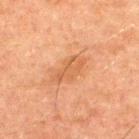The lesion was tiled from a total-body skin photograph and was not biopsied.
Imaged with cross-polarized lighting.
The subject is a male aged 48 to 52.
On the upper back.
Approximately 4 mm at its widest.
A 15 mm close-up extracted from a 3D total-body photography capture.